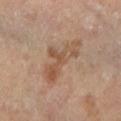<lesion>
  <biopsy_status>not biopsied; imaged during a skin examination</biopsy_status>
  <site>right leg</site>
  <image>
    <source>total-body photography crop</source>
    <field_of_view_mm>15</field_of_view_mm>
  </image>
  <lighting>cross-polarized</lighting>
  <lesion_size>
    <long_diameter_mm_approx>6.5</long_diameter_mm_approx>
  </lesion_size>
  <patient>
    <sex>female</sex>
    <age_approx>65</age_approx>
  </patient>
</lesion>